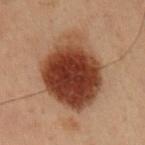Image and clinical context:
The lesion's longest dimension is about 7.5 mm. The subject is a male aged 53–57. The lesion is on the right upper arm. Cropped from a whole-body photographic skin survey; the tile spans about 15 mm. The lesion-visualizer software estimated a mean CIELAB color near L≈32 a*≈20 b*≈26, a lesion–skin lightness drop of about 16, and a normalized lesion–skin contrast near 13.5. The analysis additionally found internal color variation of about 7 on a 0–10 scale. The tile uses cross-polarized illumination.